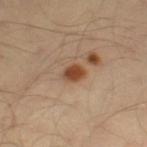This lesion was catalogued during total-body skin photography and was not selected for biopsy.